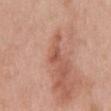{
  "lighting": "white-light",
  "automated_metrics": {
    "border_irregularity_0_10": 3.5,
    "color_variation_0_10": 1.0,
    "peripheral_color_asymmetry": 0.5
  },
  "site": "mid back",
  "lesion_size": {
    "long_diameter_mm_approx": 2.5
  },
  "patient": {
    "sex": "female",
    "age_approx": 40
  },
  "image": {
    "source": "total-body photography crop",
    "field_of_view_mm": 15
  }
}